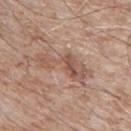Part of a total-body skin-imaging series; this lesion was reviewed on a skin check and was not flagged for biopsy. Imaged with white-light lighting. On the chest. A region of skin cropped from a whole-body photographic capture, roughly 15 mm wide. The patient is a male about 65 years old.